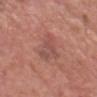{
  "biopsy_status": "not biopsied; imaged during a skin examination",
  "image": {
    "source": "total-body photography crop",
    "field_of_view_mm": 15
  },
  "lesion_size": {
    "long_diameter_mm_approx": 4.5
  },
  "automated_metrics": {
    "area_mm2_approx": 9.5,
    "eccentricity": 0.8,
    "shape_asymmetry": 0.35
  },
  "patient": {
    "sex": "male",
    "age_approx": 60
  },
  "site": "head or neck"
}